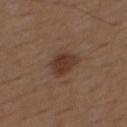Q: Was a biopsy performed?
A: imaged on a skin check; not biopsied
Q: Lesion location?
A: the mid back
Q: Patient demographics?
A: male, aged 73 to 77
Q: How large is the lesion?
A: ~3.5 mm (longest diameter)
Q: How was the tile lit?
A: white-light
Q: What is the imaging modality?
A: 15 mm crop, total-body photography